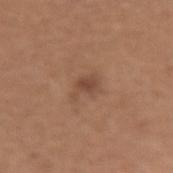Impression: Imaged during a routine full-body skin examination; the lesion was not biopsied and no histopathology is available. Acquisition and patient details: The subject is a female in their 50s. Located on the right upper arm. This image is a 15 mm lesion crop taken from a total-body photograph. Automated tile analysis of the lesion measured a border-irregularity rating of about 4/10, internal color variation of about 1.5 on a 0–10 scale, and radial color variation of about 0.5. About 3 mm across.